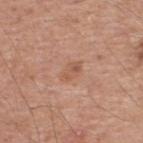workup: total-body-photography surveillance lesion; no biopsy
illumination: white-light illumination
size: about 3 mm
patient: male, in their mid-50s
image-analysis metrics: an eccentricity of roughly 0.8 and a shape-asymmetry score of about 0.2 (0 = symmetric); a classifier nevus-likeness of about 10/100
body site: the upper back
image source: total-body-photography crop, ~15 mm field of view